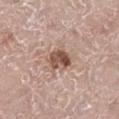workup — imaged on a skin check; not biopsied | site — the right leg | subject — male, aged around 70 | illumination — white-light illumination | TBP lesion metrics — a lesion area of about 8 mm², an outline eccentricity of about 0.75 (0 = round, 1 = elongated), and a symmetry-axis asymmetry near 0.25; a border-irregularity index near 3/10, internal color variation of about 6.5 on a 0–10 scale, and radial color variation of about 2.5; an automated nevus-likeness rating near 90 out of 100 | imaging modality — total-body-photography crop, ~15 mm field of view.